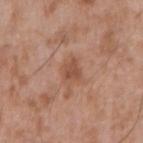Q: Was this lesion biopsied?
A: catalogued during a skin exam; not biopsied
Q: Illumination type?
A: white-light illumination
Q: What kind of image is this?
A: ~15 mm crop, total-body skin-cancer survey
Q: Where on the body is the lesion?
A: the right upper arm
Q: What are the patient's age and sex?
A: male, aged 48–52
Q: How large is the lesion?
A: ≈3 mm